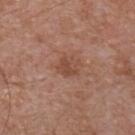Image and clinical context: A male subject, aged around 55. Captured under white-light illumination. The lesion-visualizer software estimated a footprint of about 4 mm² and an outline eccentricity of about 0.6 (0 = round, 1 = elongated). It also reported a border-irregularity index near 2.5/10, a color-variation rating of about 1.5/10, and a peripheral color-asymmetry measure near 0.5. It also reported a detector confidence of about 100 out of 100 that the crop contains a lesion. The lesion is on the chest. A lesion tile, about 15 mm wide, cut from a 3D total-body photograph. Longest diameter approximately 2.5 mm.